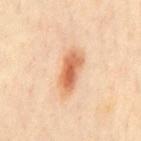Context: The lesion is on the chest. A male subject aged 48–52. Approximately 5 mm at its widest. The lesion-visualizer software estimated a footprint of about 9.5 mm², an eccentricity of roughly 0.9, and a symmetry-axis asymmetry near 0.25. And it measured an average lesion color of about L≈66 a*≈25 b*≈38 (CIELAB), about 14 CIELAB-L* units darker than the surrounding skin, and a lesion-to-skin contrast of about 9 (normalized; higher = more distinct). The analysis additionally found a border-irregularity rating of about 3/10, a color-variation rating of about 6/10, and a peripheral color-asymmetry measure near 2. A lesion tile, about 15 mm wide, cut from a 3D total-body photograph. The tile uses cross-polarized illumination.